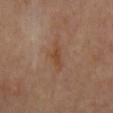The lesion is located on the mid back.
Captured under cross-polarized illumination.
A close-up tile cropped from a whole-body skin photograph, about 15 mm across.